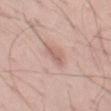A male patient, about 40 years old. Approximately 3 mm at its widest. A 15 mm crop from a total-body photograph taken for skin-cancer surveillance. Imaged with white-light lighting. On the left thigh.